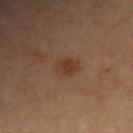follow-up = total-body-photography surveillance lesion; no biopsy
subject = female, about 40 years old
image-analysis metrics = an area of roughly 5.5 mm², an eccentricity of roughly 0.55, and a symmetry-axis asymmetry near 0.15; a border-irregularity index near 1.5/10 and a within-lesion color-variation index near 2/10; an automated nevus-likeness rating near 75 out of 100 and a detector confidence of about 100 out of 100 that the crop contains a lesion
image source = ~15 mm crop, total-body skin-cancer survey
location = the right forearm
lighting = cross-polarized illumination